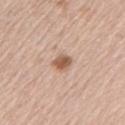| feature | finding |
|---|---|
| follow-up | imaged on a skin check; not biopsied |
| subject | male, in their mid-60s |
| TBP lesion metrics | an eccentricity of roughly 0.65 and a symmetry-axis asymmetry near 0.25; a lesion color around L≈57 a*≈21 b*≈31 in CIELAB, roughly 14 lightness units darker than nearby skin, and a normalized lesion–skin contrast near 9.5; a nevus-likeness score of about 95/100 and lesion-presence confidence of about 100/100 |
| size | about 2.5 mm |
| lighting | white-light |
| body site | the left upper arm |
| imaging modality | 15 mm crop, total-body photography |